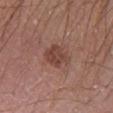The lesion was tiled from a total-body skin photograph and was not biopsied. Measured at roughly 3.5 mm in maximum diameter. Automated image analysis of the tile measured an area of roughly 7.5 mm², an eccentricity of roughly 0.6, and a symmetry-axis asymmetry near 0.2. And it measured a border-irregularity rating of about 2.5/10 and a within-lesion color-variation index near 3/10. A 15 mm close-up tile from a total-body photography series done for melanoma screening. On the left lower leg. A male patient aged 18 to 22. The tile uses white-light illumination.